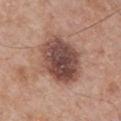Impression: No biopsy was performed on this lesion — it was imaged during a full skin examination and was not determined to be concerning. Clinical summary: A 15 mm crop from a total-body photograph taken for skin-cancer surveillance. From the chest. The total-body-photography lesion software estimated an average lesion color of about L≈47 a*≈20 b*≈24 (CIELAB), roughly 15 lightness units darker than nearby skin, and a normalized lesion–skin contrast near 11. The software also gave a border-irregularity rating of about 1.5/10, a within-lesion color-variation index near 6.5/10, and peripheral color asymmetry of about 2. The software also gave an automated nevus-likeness rating near 15 out of 100 and a detector confidence of about 100 out of 100 that the crop contains a lesion. A male patient aged approximately 30. Measured at roughly 6.5 mm in maximum diameter. This is a white-light tile.